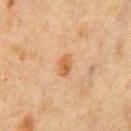Clinical summary:
A male patient, aged around 75. Cropped from a whole-body photographic skin survey; the tile spans about 15 mm. Located on the front of the torso.